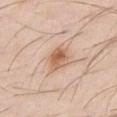Impression: The lesion was tiled from a total-body skin photograph and was not biopsied. Background: The lesion's longest dimension is about 3.5 mm. The tile uses white-light illumination. Automated tile analysis of the lesion measured a lesion color around L≈62 a*≈21 b*≈32 in CIELAB, roughly 12 lightness units darker than nearby skin, and a lesion-to-skin contrast of about 8 (normalized; higher = more distinct). The software also gave a classifier nevus-likeness of about 90/100 and lesion-presence confidence of about 100/100. From the chest. Cropped from a total-body skin-imaging series; the visible field is about 15 mm. The patient is a male aged approximately 30.